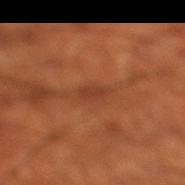Image and clinical context:
Located on the right thigh. A 15 mm crop from a total-body photograph taken for skin-cancer surveillance. A male patient aged approximately 70. The tile uses cross-polarized illumination. The recorded lesion diameter is about 3 mm.